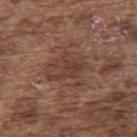| feature | finding |
|---|---|
| workup | total-body-photography surveillance lesion; no biopsy |
| patient | male, about 75 years old |
| location | the upper back |
| acquisition | ~15 mm tile from a whole-body skin photo |
| illumination | white-light |
| lesion size | ~4.5 mm (longest diameter) |